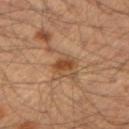The lesion was tiled from a total-body skin photograph and was not biopsied. A male patient, aged around 35. The tile uses cross-polarized illumination. About 2.5 mm across. This image is a 15 mm lesion crop taken from a total-body photograph. Located on the right forearm.